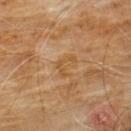The lesion was tiled from a total-body skin photograph and was not biopsied. A male patient aged 58 to 62. A roughly 15 mm field-of-view crop from a total-body skin photograph. From the chest.